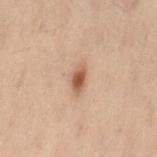Case summary:
- workup · catalogued during a skin exam; not biopsied
- body site · the lower back
- image · ~15 mm crop, total-body skin-cancer survey
- subject · female, roughly 55 years of age